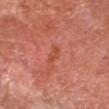Findings:
* follow-up — total-body-photography surveillance lesion; no biopsy
* site — the right forearm
* patient — male, in their 70s
* diameter — ≈2.5 mm
* lighting — cross-polarized
* image — 15 mm crop, total-body photography